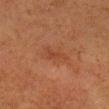<tbp_lesion>
  <biopsy_status>not biopsied; imaged during a skin examination</biopsy_status>
  <patient>
    <sex>female</sex>
    <age_approx>50</age_approx>
  </patient>
  <automated_metrics>
    <area_mm2_approx>3.0</area_mm2_approx>
    <cielab_L>35</cielab_L>
    <cielab_a>23</cielab_a>
    <cielab_b>29</cielab_b>
    <vs_skin_darker_L>5.0</vs_skin_darker_L>
    <vs_skin_contrast_norm>5.0</vs_skin_contrast_norm>
    <border_irregularity_0_10>4.5</border_irregularity_0_10>
    <color_variation_0_10>0.5</color_variation_0_10>
    <peripheral_color_asymmetry>0.0</peripheral_color_asymmetry>
    <nevus_likeness_0_100>0</nevus_likeness_0_100>
    <lesion_detection_confidence_0_100>100</lesion_detection_confidence_0_100>
  </automated_metrics>
  <site>head or neck</site>
  <image>
    <source>total-body photography crop</source>
    <field_of_view_mm>15</field_of_view_mm>
  </image>
  <lighting>cross-polarized</lighting>
</tbp_lesion>